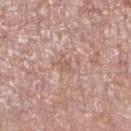Assessment:
This lesion was catalogued during total-body skin photography and was not selected for biopsy.
Context:
A 15 mm close-up tile from a total-body photography series done for melanoma screening. The lesion is located on the leg. The patient is a female aged approximately 75.